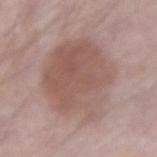Impression: Part of a total-body skin-imaging series; this lesion was reviewed on a skin check and was not flagged for biopsy. Clinical summary: The lesion is on the right forearm. About 8.5 mm across. The tile uses white-light illumination. A 15 mm close-up tile from a total-body photography series done for melanoma screening. The lesion-visualizer software estimated a footprint of about 46 mm², an outline eccentricity of about 0.55 (0 = round, 1 = elongated), and a symmetry-axis asymmetry near 0.15. The analysis additionally found a border-irregularity rating of about 2.5/10, a color-variation rating of about 4/10, and a peripheral color-asymmetry measure near 1.5. And it measured a nevus-likeness score of about 15/100 and a lesion-detection confidence of about 100/100. A male patient aged 68 to 72.